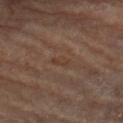<record>
<biopsy_status>not biopsied; imaged during a skin examination</biopsy_status>
<site>left thigh</site>
<automated_metrics>
  <area_mm2_approx>2.5</area_mm2_approx>
  <eccentricity>0.9</eccentricity>
  <shape_asymmetry>0.3</shape_asymmetry>
  <vs_skin_darker_L>4.0</vs_skin_darker_L>
  <vs_skin_contrast_norm>5.5</vs_skin_contrast_norm>
  <nevus_likeness_0_100>0</nevus_likeness_0_100>
  <lesion_detection_confidence_0_100>65</lesion_detection_confidence_0_100>
</automated_metrics>
<patient>
  <sex>male</sex>
  <age_approx>85+</age_approx>
</patient>
<lighting>cross-polarized</lighting>
<lesion_size>
  <long_diameter_mm_approx>2.5</long_diameter_mm_approx>
</lesion_size>
<image>
  <source>total-body photography crop</source>
  <field_of_view_mm>15</field_of_view_mm>
</image>
</record>